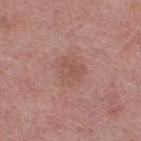Q: Was a biopsy performed?
A: total-body-photography surveillance lesion; no biopsy
Q: Patient demographics?
A: female, approximately 70 years of age
Q: What is the imaging modality?
A: ~15 mm tile from a whole-body skin photo
Q: Where on the body is the lesion?
A: the right thigh